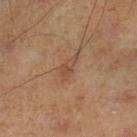follow-up: total-body-photography surveillance lesion; no biopsy
automated lesion analysis: an area of roughly 3.5 mm², an outline eccentricity of about 0.9 (0 = round, 1 = elongated), and a shape-asymmetry score of about 0.4 (0 = symmetric); border irregularity of about 4.5 on a 0–10 scale, internal color variation of about 1 on a 0–10 scale, and radial color variation of about 0; an automated nevus-likeness rating near 0 out of 100 and lesion-presence confidence of about 100/100
illumination: cross-polarized
patient: male, aged 43 to 47
image source: 15 mm crop, total-body photography
body site: the left lower leg
lesion diameter: ~3 mm (longest diameter)